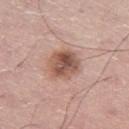{"biopsy_status": "not biopsied; imaged during a skin examination", "patient": {"sex": "male", "age_approx": 70}, "site": "leg", "automated_metrics": {"eccentricity": 0.2, "cielab_L": 54, "cielab_a": 21, "cielab_b": 27, "vs_skin_darker_L": 13.0, "vs_skin_contrast_norm": 9.0, "border_irregularity_0_10": 2.0, "color_variation_0_10": 7.0, "peripheral_color_asymmetry": 2.5, "nevus_likeness_0_100": 40, "lesion_detection_confidence_0_100": 100}, "lighting": "white-light", "image": {"source": "total-body photography crop", "field_of_view_mm": 15}}